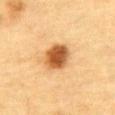notes: total-body-photography surveillance lesion; no biopsy
TBP lesion metrics: a lesion color around L≈50 a*≈22 b*≈39 in CIELAB, about 16 CIELAB-L* units darker than the surrounding skin, and a lesion-to-skin contrast of about 11 (normalized; higher = more distinct)
body site: the front of the torso
image: ~15 mm tile from a whole-body skin photo
subject: male, in their mid- to late 80s or older
lighting: cross-polarized